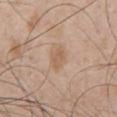Captured during whole-body skin photography for melanoma surveillance; the lesion was not biopsied. A roughly 15 mm field-of-view crop from a total-body skin photograph. The patient is a male aged approximately 50. On the chest. This is a white-light tile. Automated tile analysis of the lesion measured a shape-asymmetry score of about 0.3 (0 = symmetric). The lesion's longest dimension is about 2.5 mm.